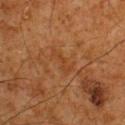notes: no biopsy performed (imaged during a skin exam) | size: ≈3.5 mm | image-analysis metrics: a footprint of about 3.5 mm² and a shape-asymmetry score of about 0.5 (0 = symmetric); a classifier nevus-likeness of about 0/100 and a lesion-detection confidence of about 95/100 | body site: the upper back | patient: male, roughly 60 years of age | acquisition: total-body-photography crop, ~15 mm field of view.